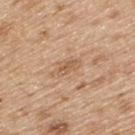The lesion is located on the back.
Cropped from a whole-body photographic skin survey; the tile spans about 15 mm.
The lesion's longest dimension is about 3.5 mm.
An algorithmic analysis of the crop reported a mean CIELAB color near L≈59 a*≈19 b*≈33, roughly 8 lightness units darker than nearby skin, and a lesion-to-skin contrast of about 5.5 (normalized; higher = more distinct). And it measured border irregularity of about 2.5 on a 0–10 scale and a color-variation rating of about 2/10. The analysis additionally found a nevus-likeness score of about 5/100 and a lesion-detection confidence of about 100/100.
The tile uses white-light illumination.
The patient is a male in their 70s.